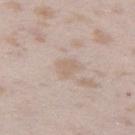lesion diameter = about 2.5 mm
tile lighting = white-light
anatomic site = the left thigh
patient = female, aged approximately 25
image = total-body-photography crop, ~15 mm field of view